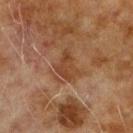Assessment:
The lesion was tiled from a total-body skin photograph and was not biopsied.
Image and clinical context:
Longest diameter approximately 3.5 mm. A 15 mm close-up extracted from a 3D total-body photography capture. Imaged with cross-polarized lighting. A male subject aged approximately 70. The lesion is on the arm.